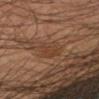Captured during whole-body skin photography for melanoma surveillance; the lesion was not biopsied.
Cropped from a total-body skin-imaging series; the visible field is about 15 mm.
Automated image analysis of the tile measured a lesion area of about 8 mm², a shape eccentricity near 0.7, and two-axis asymmetry of about 0.25. The analysis additionally found about 6 CIELAB-L* units darker than the surrounding skin. The analysis additionally found an automated nevus-likeness rating near 0 out of 100 and lesion-presence confidence of about 80/100.
Measured at roughly 4 mm in maximum diameter.
The tile uses cross-polarized illumination.
A male subject, aged 33–37.
On the right forearm.